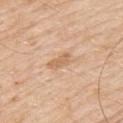follow-up = imaged on a skin check; not biopsied
subject = male, aged approximately 60
image = 15 mm crop, total-body photography
lighting = white-light illumination
location = the left upper arm